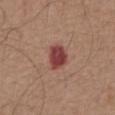Acquisition and patient details: The tile uses white-light illumination. This image is a 15 mm lesion crop taken from a total-body photograph. The lesion is on the abdomen. The subject is a male aged 73–77. The lesion's longest dimension is about 3 mm.